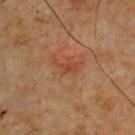Notes:
• size — ~3 mm (longest diameter)
• body site — the chest
• illumination — cross-polarized
• subject — male, aged 63 to 67
• acquisition — 15 mm crop, total-body photography